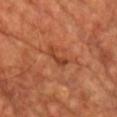Clinical impression: No biopsy was performed on this lesion — it was imaged during a full skin examination and was not determined to be concerning. Context: Measured at roughly 3 mm in maximum diameter. A male patient, approximately 60 years of age. A 15 mm close-up extracted from a 3D total-body photography capture. The lesion is located on the chest. Imaged with cross-polarized lighting.